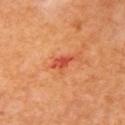Assessment: Recorded during total-body skin imaging; not selected for excision or biopsy. Acquisition and patient details: A female subject aged approximately 65. The tile uses cross-polarized illumination. The total-body-photography lesion software estimated a footprint of about 3 mm², an eccentricity of roughly 0.85, and two-axis asymmetry of about 0.25. The analysis additionally found a lesion color around L≈50 a*≈38 b*≈38 in CIELAB. And it measured internal color variation of about 2.5 on a 0–10 scale and a peripheral color-asymmetry measure near 1. The analysis additionally found a classifier nevus-likeness of about 0/100 and a detector confidence of about 100 out of 100 that the crop contains a lesion. From the right upper arm. A roughly 15 mm field-of-view crop from a total-body skin photograph. Approximately 2.5 mm at its widest.